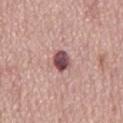| field | value |
|---|---|
| notes | catalogued during a skin exam; not biopsied |
| TBP lesion metrics | a footprint of about 5 mm² and a symmetry-axis asymmetry near 0.25; a mean CIELAB color near L≈48 a*≈24 b*≈17, roughly 18 lightness units darker than nearby skin, and a normalized lesion–skin contrast near 13; a border-irregularity rating of about 2/10, internal color variation of about 5.5 on a 0–10 scale, and peripheral color asymmetry of about 1.5; a nevus-likeness score of about 45/100 and a detector confidence of about 100 out of 100 that the crop contains a lesion |
| image | 15 mm crop, total-body photography |
| size | ~2.5 mm (longest diameter) |
| location | the mid back |
| illumination | white-light illumination |
| subject | male, about 75 years old |